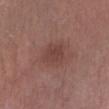image source: total-body-photography crop, ~15 mm field of view; diameter: ~3 mm (longest diameter); tile lighting: white-light illumination; patient: female, aged approximately 50; anatomic site: the left lower leg.